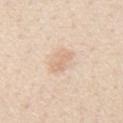Clinical impression: Part of a total-body skin-imaging series; this lesion was reviewed on a skin check and was not flagged for biopsy. Image and clinical context: The lesion is on the mid back. A male patient, aged approximately 60. A region of skin cropped from a whole-body photographic capture, roughly 15 mm wide.